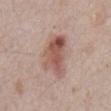• follow-up — no biopsy performed (imaged during a skin exam)
• subject — male, in their mid-60s
• imaging modality — 15 mm crop, total-body photography
• site — the chest
• size — ≈5.5 mm